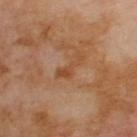This is a cross-polarized tile. A 15 mm crop from a total-body photograph taken for skin-cancer surveillance. Located on the upper back. The recorded lesion diameter is about 4 mm. Automated tile analysis of the lesion measured a footprint of about 3.5 mm², an outline eccentricity of about 0.95 (0 = round, 1 = elongated), and two-axis asymmetry of about 0.55. The analysis additionally found a lesion color around L≈46 a*≈22 b*≈34 in CIELAB and a normalized border contrast of about 6. And it measured a border-irregularity index near 7/10, a within-lesion color-variation index near 0.5/10, and radial color variation of about 0. The analysis additionally found a nevus-likeness score of about 0/100 and a detector confidence of about 100 out of 100 that the crop contains a lesion. A male subject aged 68–72.